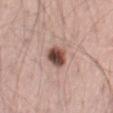biopsy status: imaged on a skin check; not biopsied | tile lighting: white-light illumination | imaging modality: ~15 mm crop, total-body skin-cancer survey | diameter: about 3 mm | patient: male, about 40 years old | location: the leg.